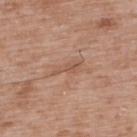About 3.5 mm across. A 15 mm close-up extracted from a 3D total-body photography capture. A male subject aged around 50. From the upper back.